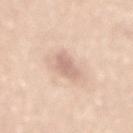Assessment: The lesion was photographed on a routine skin check and not biopsied; there is no pathology result. Background: Approximately 3 mm at its widest. Captured under white-light illumination. The patient is a male aged approximately 50. From the lower back. A 15 mm crop from a total-body photograph taken for skin-cancer surveillance.